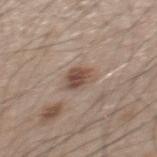The lesion was photographed on a routine skin check and not biopsied; there is no pathology result. Measured at roughly 3 mm in maximum diameter. A male subject aged approximately 50. Cropped from a total-body skin-imaging series; the visible field is about 15 mm. The lesion is on the mid back. Captured under white-light illumination. Automated image analysis of the tile measured an area of roughly 5.5 mm² and an outline eccentricity of about 0.55 (0 = round, 1 = elongated). The analysis additionally found a classifier nevus-likeness of about 80/100 and lesion-presence confidence of about 100/100.